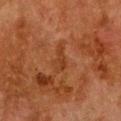Captured under cross-polarized illumination. The patient is a female approximately 50 years of age. From the chest. This image is a 15 mm lesion crop taken from a total-body photograph. Approximately 3.5 mm at its widest.